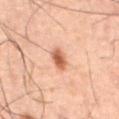Recorded during total-body skin imaging; not selected for excision or biopsy. A roughly 15 mm field-of-view crop from a total-body skin photograph. This is a cross-polarized tile. Longest diameter approximately 2.5 mm. A male patient roughly 60 years of age. Located on the abdomen.